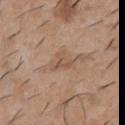Q: Is there a histopathology result?
A: catalogued during a skin exam; not biopsied
Q: How was this image acquired?
A: ~15 mm tile from a whole-body skin photo
Q: Automated lesion metrics?
A: a mean CIELAB color near L≈53 a*≈17 b*≈29, roughly 7 lightness units darker than nearby skin, and a normalized lesion–skin contrast near 5; a border-irregularity rating of about 5.5/10
Q: Lesion location?
A: the front of the torso
Q: What lighting was used for the tile?
A: white-light illumination
Q: What is the lesion's diameter?
A: ~2.5 mm (longest diameter)
Q: Patient demographics?
A: male, about 40 years old